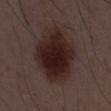Imaged during a routine full-body skin examination; the lesion was not biopsied and no histopathology is available. A 15 mm close-up tile from a total-body photography series done for melanoma screening. The tile uses white-light illumination. A male patient about 50 years old. Approximately 8.5 mm at its widest.